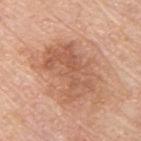{
  "patient": {
    "sex": "male",
    "age_approx": 80
  },
  "lesion_size": {
    "long_diameter_mm_approx": 7.0
  },
  "site": "back",
  "image": {
    "source": "total-body photography crop",
    "field_of_view_mm": 15
  }
}